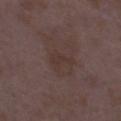Recorded during total-body skin imaging; not selected for excision or biopsy. The lesion's longest dimension is about 3 mm. The patient is a female aged 33 to 37. Cropped from a whole-body photographic skin survey; the tile spans about 15 mm. An algorithmic analysis of the crop reported an eccentricity of roughly 0.75 and a shape-asymmetry score of about 0.45 (0 = symmetric). And it measured an average lesion color of about L≈33 a*≈15 b*≈19 (CIELAB) and a lesion-to-skin contrast of about 5 (normalized; higher = more distinct). The analysis additionally found an automated nevus-likeness rating near 0 out of 100 and lesion-presence confidence of about 100/100. Captured under white-light illumination. From the left thigh.